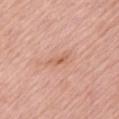notes: imaged on a skin check; not biopsied | illumination: white-light illumination | size: ≈2.5 mm | acquisition: ~15 mm crop, total-body skin-cancer survey | patient: male, aged 73 to 77 | anatomic site: the left upper arm.